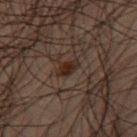Q: Was a biopsy performed?
A: imaged on a skin check; not biopsied
Q: How was the tile lit?
A: cross-polarized illumination
Q: What are the patient's age and sex?
A: male, aged 48 to 52
Q: What is the anatomic site?
A: the right thigh
Q: How was this image acquired?
A: total-body-photography crop, ~15 mm field of view
Q: How large is the lesion?
A: ≈2.5 mm
Q: What did automated image analysis measure?
A: a mean CIELAB color near L≈18 a*≈12 b*≈17; an automated nevus-likeness rating near 65 out of 100 and a detector confidence of about 100 out of 100 that the crop contains a lesion